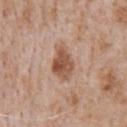workup: total-body-photography surveillance lesion; no biopsy | patient: male, in their mid-60s | anatomic site: the chest | acquisition: 15 mm crop, total-body photography | automated metrics: an area of roughly 9 mm² and two-axis asymmetry of about 0.2.